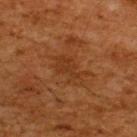Recorded during total-body skin imaging; not selected for excision or biopsy. The tile uses cross-polarized illumination. Longest diameter approximately 4.5 mm. The total-body-photography lesion software estimated a mean CIELAB color near L≈29 a*≈20 b*≈30 and a normalized border contrast of about 5.5. The analysis additionally found a border-irregularity rating of about 5.5/10, a within-lesion color-variation index near 2/10, and radial color variation of about 0.5. The analysis additionally found an automated nevus-likeness rating near 0 out of 100 and a detector confidence of about 100 out of 100 that the crop contains a lesion. A 15 mm close-up tile from a total-body photography series done for melanoma screening. A male patient in their mid-60s. The lesion is located on the upper back.